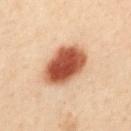- follow-up · no biopsy performed (imaged during a skin exam)
- patient · female, approximately 30 years of age
- lesion size · about 6.5 mm
- automated metrics · a lesion area of about 19 mm², a shape eccentricity near 0.8, and a shape-asymmetry score of about 0.15 (0 = symmetric); a border-irregularity index near 1.5/10, a within-lesion color-variation index near 6/10, and a peripheral color-asymmetry measure near 1.5; a nevus-likeness score of about 100/100 and a detector confidence of about 100 out of 100 that the crop contains a lesion
- body site · the mid back
- illumination · cross-polarized
- image source · 15 mm crop, total-body photography